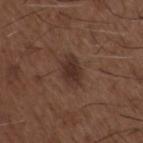Part of a total-body skin-imaging series; this lesion was reviewed on a skin check and was not flagged for biopsy. On the chest. A male subject aged approximately 50. The recorded lesion diameter is about 3.5 mm. A close-up tile cropped from a whole-body skin photograph, about 15 mm across. The total-body-photography lesion software estimated an average lesion color of about L≈31 a*≈18 b*≈22 (CIELAB), roughly 8 lightness units darker than nearby skin, and a normalized lesion–skin contrast near 8.5.